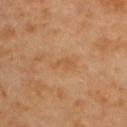| feature | finding |
|---|---|
| biopsy status | no biopsy performed (imaged during a skin exam) |
| acquisition | ~15 mm crop, total-body skin-cancer survey |
| location | the upper back |
| subject | female, aged 58–62 |